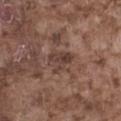<record>
<biopsy_status>not biopsied; imaged during a skin examination</biopsy_status>
<patient>
  <sex>male</sex>
  <age_approx>75</age_approx>
</patient>
<image>
  <source>total-body photography crop</source>
  <field_of_view_mm>15</field_of_view_mm>
</image>
<site>left thigh</site>
</record>